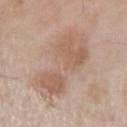Recorded during total-body skin imaging; not selected for excision or biopsy. A roughly 15 mm field-of-view crop from a total-body skin photograph. The lesion is located on the left upper arm. A male subject in their mid-50s.